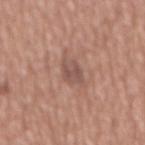The lesion's longest dimension is about 3 mm. The total-body-photography lesion software estimated a lesion area of about 4.5 mm², a shape eccentricity near 0.8, and two-axis asymmetry of about 0.25. The software also gave a border-irregularity rating of about 3/10, internal color variation of about 2 on a 0–10 scale, and peripheral color asymmetry of about 1. A male subject, roughly 45 years of age. Cropped from a total-body skin-imaging series; the visible field is about 15 mm. On the front of the torso.